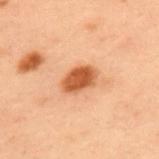Findings:
• biopsy status · catalogued during a skin exam; not biopsied
• automated lesion analysis · a border-irregularity index near 1.5/10, a color-variation rating of about 3.5/10, and a peripheral color-asymmetry measure near 1
• patient · male, in their mid- to late 50s
• lesion size · ~4 mm (longest diameter)
• image · ~15 mm tile from a whole-body skin photo
• location · the upper back